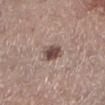Case summary:
- notes · catalogued during a skin exam; not biopsied
- lighting · white-light
- image · total-body-photography crop, ~15 mm field of view
- location · the left lower leg
- automated metrics · a footprint of about 5.5 mm², a shape eccentricity near 0.6, and two-axis asymmetry of about 0.3; a lesion color around L≈48 a*≈17 b*≈21 in CIELAB, a lesion–skin lightness drop of about 14, and a lesion-to-skin contrast of about 10 (normalized; higher = more distinct); a color-variation rating of about 4/10 and a peripheral color-asymmetry measure near 1; a classifier nevus-likeness of about 95/100 and lesion-presence confidence of about 100/100
- subject · male, aged 53 to 57
- diameter · ~3 mm (longest diameter)